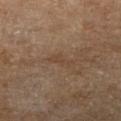Imaged during a routine full-body skin examination; the lesion was not biopsied and no histopathology is available. Located on the right lower leg. The subject is a male aged 83 or over. Imaged with cross-polarized lighting. Longest diameter approximately 3 mm. A 15 mm crop from a total-body photograph taken for skin-cancer surveillance.